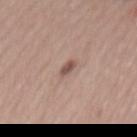follow-up=imaged on a skin check; not biopsied | TBP lesion metrics=an average lesion color of about L≈52 a*≈18 b*≈24 (CIELAB) and a normalized border contrast of about 8 | image=total-body-photography crop, ~15 mm field of view | site=the mid back | patient=female, aged approximately 55 | size=about 2.5 mm | illumination=white-light.